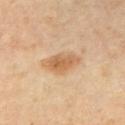Clinical impression:
Imaged during a routine full-body skin examination; the lesion was not biopsied and no histopathology is available.
Image and clinical context:
A 15 mm close-up extracted from a 3D total-body photography capture. Captured under cross-polarized illumination. Measured at roughly 3.5 mm in maximum diameter. The lesion is located on the chest. A female patient in their 40s.